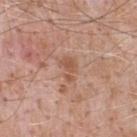| key | value |
|---|---|
| biopsy status | total-body-photography surveillance lesion; no biopsy |
| patient | male, about 50 years old |
| illumination | white-light illumination |
| image-analysis metrics | an area of roughly 4.5 mm² and a symmetry-axis asymmetry near 0.4; a mean CIELAB color near L≈54 a*≈21 b*≈30 and a normalized lesion–skin contrast near 6.5 |
| image source | total-body-photography crop, ~15 mm field of view |
| site | the upper back |